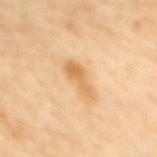Findings:
• follow-up — catalogued during a skin exam; not biopsied
• diameter — ≈4 mm
• imaging modality — 15 mm crop, total-body photography
• patient — female, aged approximately 45
• illumination — cross-polarized illumination
• location — the upper back